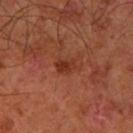follow-up = imaged on a skin check; not biopsied | patient = male, aged 58–62 | location = the left forearm | lesion size = about 3 mm | acquisition = ~15 mm tile from a whole-body skin photo.